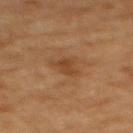Impression:
This lesion was catalogued during total-body skin photography and was not selected for biopsy.
Acquisition and patient details:
The lesion-visualizer software estimated a shape eccentricity near 0.6 and a symmetry-axis asymmetry near 0.4. It also reported an average lesion color of about L≈38 a*≈19 b*≈31 (CIELAB), a lesion–skin lightness drop of about 7, and a normalized lesion–skin contrast near 6. The software also gave a classifier nevus-likeness of about 20/100 and a lesion-detection confidence of about 100/100. The lesion is located on the upper back. The patient is a female approximately 55 years of age. The lesion's longest dimension is about 3 mm. A lesion tile, about 15 mm wide, cut from a 3D total-body photograph.